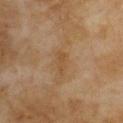Clinical impression:
Captured during whole-body skin photography for melanoma surveillance; the lesion was not biopsied.
Image and clinical context:
The tile uses cross-polarized illumination. Automated tile analysis of the lesion measured a lesion area of about 3.5 mm² and a shape-asymmetry score of about 0.3 (0 = symmetric). And it measured a border-irregularity index near 3/10 and a within-lesion color-variation index near 1.5/10. The subject is a female aged approximately 60. A 15 mm close-up tile from a total-body photography series done for melanoma screening. The lesion is on the upper back.